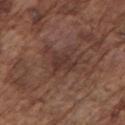{
  "biopsy_status": "not biopsied; imaged during a skin examination",
  "patient": {
    "sex": "male",
    "age_approx": 75
  },
  "site": "left upper arm",
  "automated_metrics": {
    "area_mm2_approx": 5.0,
    "shape_asymmetry": 0.3,
    "border_irregularity_0_10": 3.0,
    "color_variation_0_10": 2.5,
    "peripheral_color_asymmetry": 0.5,
    "nevus_likeness_0_100": 0,
    "lesion_detection_confidence_0_100": 95
  },
  "image": {
    "source": "total-body photography crop",
    "field_of_view_mm": 15
  }
}